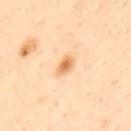Assessment: Recorded during total-body skin imaging; not selected for excision or biopsy. Acquisition and patient details: A close-up tile cropped from a whole-body skin photograph, about 15 mm across. This is a cross-polarized tile. The lesion's longest dimension is about 3 mm. A male subject approximately 45 years of age. On the upper back.